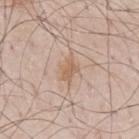This lesion was catalogued during total-body skin photography and was not selected for biopsy. Longest diameter approximately 3 mm. A male subject aged around 80. The lesion-visualizer software estimated a lesion color around L≈61 a*≈17 b*≈30 in CIELAB, roughly 8 lightness units darker than nearby skin, and a normalized lesion–skin contrast near 6. The analysis additionally found a border-irregularity index near 3.5/10, a within-lesion color-variation index near 1.5/10, and peripheral color asymmetry of about 0.5. The software also gave a nevus-likeness score of about 0/100 and a lesion-detection confidence of about 100/100. Cropped from a total-body skin-imaging series; the visible field is about 15 mm. The lesion is located on the mid back.